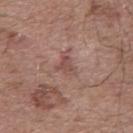Automated image analysis of the tile measured an average lesion color of about L≈49 a*≈21 b*≈23 (CIELAB) and roughly 8 lightness units darker than nearby skin.
A lesion tile, about 15 mm wide, cut from a 3D total-body photograph.
A male subject in their mid- to late 50s.
The lesion is located on the back.
The recorded lesion diameter is about 3 mm.